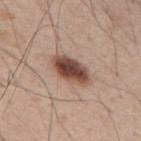subject = male, approximately 65 years of age; anatomic site = the upper back; imaging modality = total-body-photography crop, ~15 mm field of view; diameter = ≈5 mm; illumination = white-light.